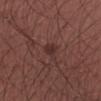The lesion was tiled from a total-body skin photograph and was not biopsied.
Measured at roughly 3.5 mm in maximum diameter.
Captured under white-light illumination.
The subject is a male aged 33 to 37.
The total-body-photography lesion software estimated a lesion area of about 3.5 mm² and an outline eccentricity of about 0.9 (0 = round, 1 = elongated). And it measured a border-irregularity index near 4.5/10, a color-variation rating of about 1/10, and radial color variation of about 0.5. The software also gave an automated nevus-likeness rating near 20 out of 100 and lesion-presence confidence of about 95/100.
The lesion is on the right forearm.
This image is a 15 mm lesion crop taken from a total-body photograph.